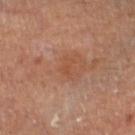{"biopsy_status": "not biopsied; imaged during a skin examination", "site": "leg", "image": {"source": "total-body photography crop", "field_of_view_mm": 15}, "lesion_size": {"long_diameter_mm_approx": 2.5}, "lighting": "cross-polarized", "automated_metrics": {"area_mm2_approx": 2.5, "eccentricity": 0.85, "cielab_L": 47, "cielab_a": 23, "cielab_b": 32, "vs_skin_darker_L": 5.0, "vs_skin_contrast_norm": 5.0, "border_irregularity_0_10": 5.0, "peripheral_color_asymmetry": 0.0, "nevus_likeness_0_100": 0}, "patient": {"sex": "male", "age_approx": "85+"}}